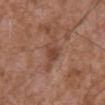Part of a total-body skin-imaging series; this lesion was reviewed on a skin check and was not flagged for biopsy. Located on the front of the torso. Longest diameter approximately 5 mm. A male patient, aged approximately 75. Imaged with white-light lighting. The lesion-visualizer software estimated a lesion area of about 6.5 mm², an outline eccentricity of about 0.9 (0 = round, 1 = elongated), and two-axis asymmetry of about 0.5. Cropped from a total-body skin-imaging series; the visible field is about 15 mm.